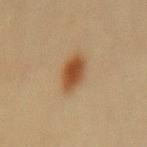  biopsy_status: not biopsied; imaged during a skin examination
  image:
    source: total-body photography crop
    field_of_view_mm: 15
  site: back
  patient:
    sex: female
    age_approx: 20